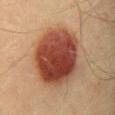Findings:
- location · the lower back
- imaging modality · ~15 mm tile from a whole-body skin photo
- patient · male, aged approximately 40